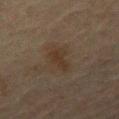The lesion was tiled from a total-body skin photograph and was not biopsied. A male patient aged approximately 70. Captured under cross-polarized illumination. A roughly 15 mm field-of-view crop from a total-body skin photograph. From the back. Approximately 3.5 mm at its widest.